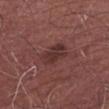{"biopsy_status": "not biopsied; imaged during a skin examination", "patient": {"sex": "male", "age_approx": 70}, "lesion_size": {"long_diameter_mm_approx": 4.0}, "automated_metrics": {"cielab_L": 33, "cielab_a": 22, "cielab_b": 19, "vs_skin_darker_L": 8.0, "vs_skin_contrast_norm": 7.5, "nevus_likeness_0_100": 20, "lesion_detection_confidence_0_100": 100}, "image": {"source": "total-body photography crop", "field_of_view_mm": 15}, "site": "right upper arm"}